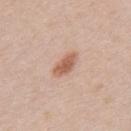notes: catalogued during a skin exam; not biopsied
image: ~15 mm tile from a whole-body skin photo
illumination: white-light
patient: male, aged 38–42
location: the upper back
automated metrics: an area of roughly 5 mm², an eccentricity of roughly 0.9, and a shape-asymmetry score of about 0.15 (0 = symmetric); a classifier nevus-likeness of about 95/100